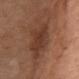Part of a total-body skin-imaging series; this lesion was reviewed on a skin check and was not flagged for biopsy.
The subject is a female aged approximately 40.
A region of skin cropped from a whole-body photographic capture, roughly 15 mm wide.
The tile uses white-light illumination.
The lesion is located on the chest.